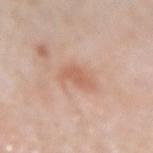Imaged during a routine full-body skin examination; the lesion was not biopsied and no histopathology is available.
The subject is a female in their mid-50s.
The lesion is located on the left forearm.
Automated tile analysis of the lesion measured a footprint of about 4.5 mm², an eccentricity of roughly 0.85, and a symmetry-axis asymmetry near 0.4. The software also gave border irregularity of about 4 on a 0–10 scale, a within-lesion color-variation index near 1/10, and a peripheral color-asymmetry measure near 0.5. The software also gave a nevus-likeness score of about 5/100 and lesion-presence confidence of about 100/100.
Captured under white-light illumination.
A 15 mm crop from a total-body photograph taken for skin-cancer surveillance.